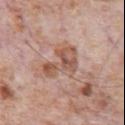– notes — total-body-photography surveillance lesion; no biopsy
– subject — male, approximately 80 years of age
– body site — the chest
– imaging modality — 15 mm crop, total-body photography